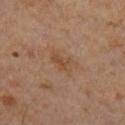Impression:
Recorded during total-body skin imaging; not selected for excision or biopsy.
Acquisition and patient details:
A male subject approximately 60 years of age. Automated tile analysis of the lesion measured a mean CIELAB color near L≈43 a*≈17 b*≈29 and a lesion-to-skin contrast of about 5.5 (normalized; higher = more distinct). The analysis additionally found a nevus-likeness score of about 0/100 and a detector confidence of about 100 out of 100 that the crop contains a lesion. The tile uses cross-polarized illumination. The lesion is on the right lower leg. This image is a 15 mm lesion crop taken from a total-body photograph.